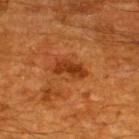Part of a total-body skin-imaging series; this lesion was reviewed on a skin check and was not flagged for biopsy. Cropped from a whole-body photographic skin survey; the tile spans about 15 mm. The lesion is located on the upper back. A male subject aged around 60.